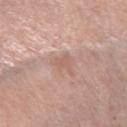<lesion>
  <biopsy_status>not biopsied; imaged during a skin examination</biopsy_status>
  <lesion_size>
    <long_diameter_mm_approx>3.0</long_diameter_mm_approx>
  </lesion_size>
  <patient>
    <sex>female</sex>
    <age_approx>65</age_approx>
  </patient>
  <image>
    <source>total-body photography crop</source>
    <field_of_view_mm>15</field_of_view_mm>
  </image>
  <site>left forearm</site>
  <automated_metrics>
    <area_mm2_approx>4.5</area_mm2_approx>
    <shape_asymmetry>0.45</shape_asymmetry>
    <cielab_L>60</cielab_L>
    <cielab_a>19</cielab_a>
    <cielab_b>26</cielab_b>
    <vs_skin_darker_L>7.0</vs_skin_darker_L>
    <vs_skin_contrast_norm>4.5</vs_skin_contrast_norm>
    <nevus_likeness_0_100>0</nevus_likeness_0_100>
    <lesion_detection_confidence_0_100>90</lesion_detection_confidence_0_100>
  </automated_metrics>
  <lighting>white-light</lighting>
</lesion>